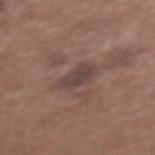notes: imaged on a skin check; not biopsied
tile lighting: white-light illumination
patient: female, about 65 years old
image: 15 mm crop, total-body photography
lesion diameter: ~3.5 mm (longest diameter)
site: the upper back
TBP lesion metrics: an area of roughly 6.5 mm² and two-axis asymmetry of about 0.25